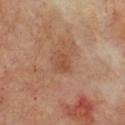Part of a total-body skin-imaging series; this lesion was reviewed on a skin check and was not flagged for biopsy. A male patient, aged approximately 70. A 15 mm close-up extracted from a 3D total-body photography capture. From the chest.